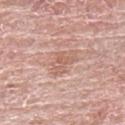No biopsy was performed on this lesion — it was imaged during a full skin examination and was not determined to be concerning. A female subject, roughly 60 years of age. The lesion is on the left upper arm. A roughly 15 mm field-of-view crop from a total-body skin photograph.